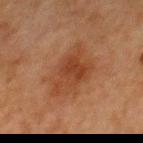Assessment:
The lesion was tiled from a total-body skin photograph and was not biopsied.
Image and clinical context:
Located on the chest. A male patient, approximately 80 years of age. A 15 mm close-up tile from a total-body photography series done for melanoma screening. Imaged with cross-polarized lighting.